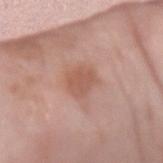Assessment: This lesion was catalogued during total-body skin photography and was not selected for biopsy. Image and clinical context: This is a white-light tile. Approximately 3.5 mm at its widest. Cropped from a total-body skin-imaging series; the visible field is about 15 mm. From the arm. A female subject aged 58 to 62. Automated image analysis of the tile measured a normalized lesion–skin contrast near 6.